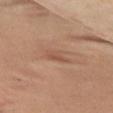No biopsy was performed on this lesion — it was imaged during a full skin examination and was not determined to be concerning.
Automated image analysis of the tile measured an area of roughly 3 mm² and a symmetry-axis asymmetry near 0.45. It also reported a border-irregularity rating of about 4.5/10, a color-variation rating of about 0/10, and a peripheral color-asymmetry measure near 0.
Measured at roughly 3 mm in maximum diameter.
A 15 mm close-up tile from a total-body photography series done for melanoma screening.
On the chest.
A female patient aged 53–57.